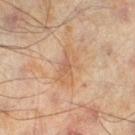Recorded during total-body skin imaging; not selected for excision or biopsy.
Located on the left lower leg.
The subject is a male approximately 45 years of age.
A region of skin cropped from a whole-body photographic capture, roughly 15 mm wide.